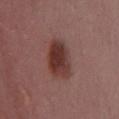Notes:
• subject · female, aged approximately 50
• location · the chest
• imaging modality · ~15 mm crop, total-body skin-cancer survey